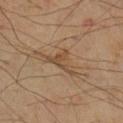workup = no biopsy performed (imaged during a skin exam); location = the right lower leg; subject = male, aged approximately 70; acquisition = ~15 mm tile from a whole-body skin photo.